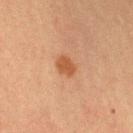No biopsy was performed on this lesion — it was imaged during a full skin examination and was not determined to be concerning. A close-up tile cropped from a whole-body skin photograph, about 15 mm across. From the left upper arm. The lesion-visualizer software estimated a lesion area of about 4 mm², an eccentricity of roughly 0.6, and two-axis asymmetry of about 0.15. It also reported internal color variation of about 1.5 on a 0–10 scale and radial color variation of about 0.5. Measured at roughly 2.5 mm in maximum diameter. A female subject, aged 53 to 57. The tile uses cross-polarized illumination.